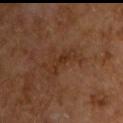This lesion was catalogued during total-body skin photography and was not selected for biopsy. This is a cross-polarized tile. The lesion is on the left upper arm. A male patient roughly 65 years of age. A 15 mm close-up tile from a total-body photography series done for melanoma screening. Longest diameter approximately 4 mm.